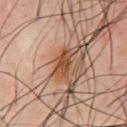| key | value |
|---|---|
| workup | imaged on a skin check; not biopsied |
| lighting | cross-polarized |
| location | the front of the torso |
| subject | male, aged 48–52 |
| image | 15 mm crop, total-body photography |